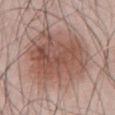Case summary:
- notes: imaged on a skin check; not biopsied
- size: ~8 mm (longest diameter)
- illumination: white-light
- anatomic site: the front of the torso
- subject: male, aged around 55
- TBP lesion metrics: a shape eccentricity near 0.4 and a symmetry-axis asymmetry near 0.2; border irregularity of about 3 on a 0–10 scale, a within-lesion color-variation index near 5.5/10, and peripheral color asymmetry of about 2
- image: 15 mm crop, total-body photography